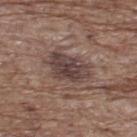Case summary:
- biopsy status: catalogued during a skin exam; not biopsied
- lighting: white-light
- subject: male, aged 68–72
- lesion diameter: ~4.5 mm (longest diameter)
- location: the upper back
- imaging modality: ~15 mm crop, total-body skin-cancer survey